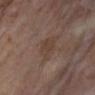Findings:
• biopsy status · imaged on a skin check; not biopsied
• illumination · cross-polarized illumination
• body site · the right lower leg
• image · 15 mm crop, total-body photography
• lesion diameter · ≈3.5 mm
• TBP lesion metrics · a footprint of about 6.5 mm², an outline eccentricity of about 0.7 (0 = round, 1 = elongated), and a shape-asymmetry score of about 0.3 (0 = symmetric)
• subject · female, aged around 55